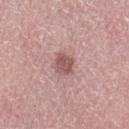The lesion was photographed on a routine skin check and not biopsied; there is no pathology result. Automated image analysis of the tile measured an area of roughly 5.5 mm², an eccentricity of roughly 0.55, and two-axis asymmetry of about 0.2. On the left thigh. This is a white-light tile. The subject is a female about 65 years old. A 15 mm close-up extracted from a 3D total-body photography capture.